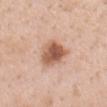Q: Was this lesion biopsied?
A: catalogued during a skin exam; not biopsied
Q: Patient demographics?
A: female, roughly 50 years of age
Q: How was this image acquired?
A: total-body-photography crop, ~15 mm field of view
Q: Lesion location?
A: the back